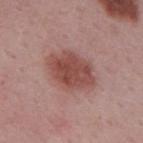biopsy_status: not biopsied; imaged during a skin examination
image:
  source: total-body photography crop
  field_of_view_mm: 15
patient:
  sex: male
  age_approx: 50
lesion_size:
  long_diameter_mm_approx: 6.0
site: back
automated_metrics:
  cielab_L: 49
  cielab_a: 24
  cielab_b: 23
  vs_skin_darker_L: 11.0
  vs_skin_contrast_norm: 8.5
  nevus_likeness_0_100: 100
lighting: white-light